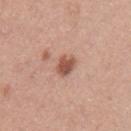Assessment: This lesion was catalogued during total-body skin photography and was not selected for biopsy. Clinical summary: Cropped from a whole-body photographic skin survey; the tile spans about 15 mm. The lesion is located on the left upper arm. The subject is a female in their mid-30s.